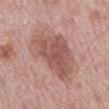The lesion was photographed on a routine skin check and not biopsied; there is no pathology result. The patient is a male in their mid- to late 70s. The lesion is on the mid back. An algorithmic analysis of the crop reported a lesion area of about 26 mm², an eccentricity of roughly 0.9, and two-axis asymmetry of about 0.3. The analysis additionally found a border-irregularity index near 4.5/10, a color-variation rating of about 3.5/10, and radial color variation of about 1. It also reported a classifier nevus-likeness of about 15/100. Captured under white-light illumination. Cropped from a total-body skin-imaging series; the visible field is about 15 mm.